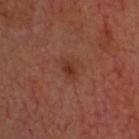<record>
  <biopsy_status>not biopsied; imaged during a skin examination</biopsy_status>
  <patient>
    <age_approx>65</age_approx>
  </patient>
  <lighting>cross-polarized</lighting>
  <site>head or neck</site>
  <image>
    <source>total-body photography crop</source>
    <field_of_view_mm>15</field_of_view_mm>
  </image>
</record>